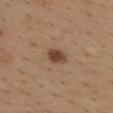This lesion was catalogued during total-body skin photography and was not selected for biopsy. A roughly 15 mm field-of-view crop from a total-body skin photograph. A female patient, about 40 years old. This is a white-light tile. The lesion is located on the upper back.